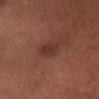Acquisition and patient details:
The lesion is located on the head or neck. The recorded lesion diameter is about 3 mm. A 15 mm crop from a total-body photograph taken for skin-cancer surveillance. A male subject aged approximately 40.